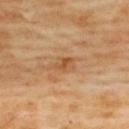The lesion was tiled from a total-body skin photograph and was not biopsied. From the upper back. The subject is a female roughly 60 years of age. This is a cross-polarized tile. A close-up tile cropped from a whole-body skin photograph, about 15 mm across. Approximately 3 mm at its widest.